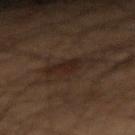Imaged during a routine full-body skin examination; the lesion was not biopsied and no histopathology is available. The total-body-photography lesion software estimated an average lesion color of about L≈18 a*≈11 b*≈17 (CIELAB), about 5 CIELAB-L* units darker than the surrounding skin, and a normalized lesion–skin contrast near 6.5. A region of skin cropped from a whole-body photographic capture, roughly 15 mm wide. The tile uses cross-polarized illumination. A male patient approximately 55 years of age. From the right forearm. About 4 mm across.